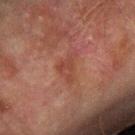• follow-up — imaged on a skin check; not biopsied
• site — the arm
• subject — male, roughly 75 years of age
• image source — ~15 mm crop, total-body skin-cancer survey
• automated lesion analysis — a footprint of about 5.5 mm², an outline eccentricity of about 0.8 (0 = round, 1 = elongated), and a shape-asymmetry score of about 0.5 (0 = symmetric); an average lesion color of about L≈36 a*≈22 b*≈24 (CIELAB) and a normalized border contrast of about 5; lesion-presence confidence of about 100/100
• diameter — about 4 mm